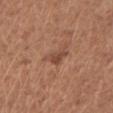Case summary:
– notes — catalogued during a skin exam; not biopsied
– subject — female, about 50 years old
– illumination — white-light illumination
– size — ~2.5 mm (longest diameter)
– site — the right forearm
– acquisition — total-body-photography crop, ~15 mm field of view
– image-analysis metrics — a footprint of about 4 mm², a shape eccentricity near 0.55, and a symmetry-axis asymmetry near 0.4; about 9 CIELAB-L* units darker than the surrounding skin and a normalized border contrast of about 6.5